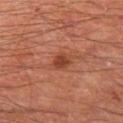<record>
<biopsy_status>not biopsied; imaged during a skin examination</biopsy_status>
</record>